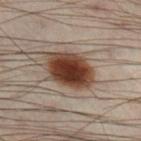Q: Was a biopsy performed?
A: imaged on a skin check; not biopsied
Q: Illumination type?
A: cross-polarized illumination
Q: Patient demographics?
A: male, approximately 50 years of age
Q: Lesion size?
A: ≈5.5 mm
Q: What is the imaging modality?
A: ~15 mm tile from a whole-body skin photo
Q: Lesion location?
A: the leg